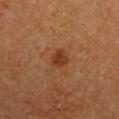Recorded during total-body skin imaging; not selected for excision or biopsy. A roughly 15 mm field-of-view crop from a total-body skin photograph. Located on the left upper arm. A male subject, aged 73–77.